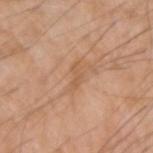No biopsy was performed on this lesion — it was imaged during a full skin examination and was not determined to be concerning.
Longest diameter approximately 3 mm.
A lesion tile, about 15 mm wide, cut from a 3D total-body photograph.
On the right upper arm.
A male patient about 65 years old.